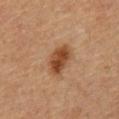{
  "biopsy_status": "not biopsied; imaged during a skin examination",
  "lesion_size": {
    "long_diameter_mm_approx": 4.0
  },
  "lighting": "cross-polarized",
  "patient": {
    "sex": "male",
    "age_approx": 60
  },
  "site": "mid back",
  "image": {
    "source": "total-body photography crop",
    "field_of_view_mm": 15
  }
}